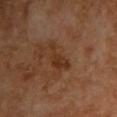Measured at roughly 4.5 mm in maximum diameter.
The lesion is on the chest.
Imaged with cross-polarized lighting.
A roughly 15 mm field-of-view crop from a total-body skin photograph.
The patient is a male approximately 60 years of age.